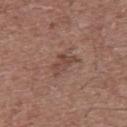| field | value |
|---|---|
| biopsy status | catalogued during a skin exam; not biopsied |
| image source | ~15 mm crop, total-body skin-cancer survey |
| body site | the upper back |
| tile lighting | white-light illumination |
| lesion diameter | ~3.5 mm (longest diameter) |
| subject | male, approximately 60 years of age |